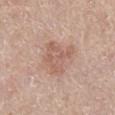Located on the left lower leg. The total-body-photography lesion software estimated a classifier nevus-likeness of about 10/100 and a lesion-detection confidence of about 100/100. A region of skin cropped from a whole-body photographic capture, roughly 15 mm wide. The patient is a female about 65 years old. Captured under white-light illumination.